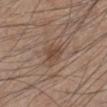Recorded during total-body skin imaging; not selected for excision or biopsy. This is a white-light tile. About 2.5 mm across. A male subject aged 43 to 47. The lesion is on the leg. A 15 mm close-up extracted from a 3D total-body photography capture. The total-body-photography lesion software estimated an area of roughly 4.5 mm² and a symmetry-axis asymmetry near 0.35. And it measured an average lesion color of about L≈45 a*≈17 b*≈26 (CIELAB) and a normalized lesion–skin contrast near 6.5. And it measured a classifier nevus-likeness of about 30/100 and a lesion-detection confidence of about 100/100.